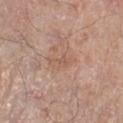Assessment:
Captured during whole-body skin photography for melanoma surveillance; the lesion was not biopsied.
Background:
Located on the right lower leg. Imaged with white-light lighting. A region of skin cropped from a whole-body photographic capture, roughly 15 mm wide. The patient is a male approximately 80 years of age. The recorded lesion diameter is about 3 mm.